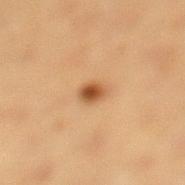notes: catalogued during a skin exam; not biopsied
image-analysis metrics: a footprint of about 4 mm², an eccentricity of roughly 0.7, and a shape-asymmetry score of about 0.25 (0 = symmetric); roughly 12 lightness units darker than nearby skin and a normalized border contrast of about 9; a nevus-likeness score of about 95/100
location: the left lower leg
subject: female, roughly 40 years of age
image source: 15 mm crop, total-body photography
diameter: ≈2.5 mm